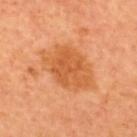Assessment:
Imaged during a routine full-body skin examination; the lesion was not biopsied and no histopathology is available.
Clinical summary:
Cropped from a whole-body photographic skin survey; the tile spans about 15 mm. A male subject roughly 65 years of age. The lesion is on the upper back.